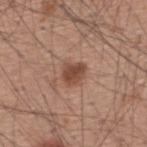Impression: Captured during whole-body skin photography for melanoma surveillance; the lesion was not biopsied. Acquisition and patient details: A male patient, about 60 years old. Located on the upper back. This is a white-light tile. Approximately 3 mm at its widest. The total-body-photography lesion software estimated a footprint of about 5.5 mm², an eccentricity of roughly 0.45, and a shape-asymmetry score of about 0.2 (0 = symmetric). It also reported border irregularity of about 2 on a 0–10 scale. A 15 mm close-up tile from a total-body photography series done for melanoma screening.